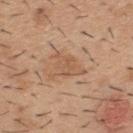| field | value |
|---|---|
| biopsy status | catalogued during a skin exam; not biopsied |
| size | about 4.5 mm |
| automated lesion analysis | an average lesion color of about L≈56 a*≈20 b*≈33 (CIELAB), a lesion–skin lightness drop of about 7, and a normalized border contrast of about 5.5; border irregularity of about 6.5 on a 0–10 scale, a color-variation rating of about 2/10, and peripheral color asymmetry of about 0.5; a lesion-detection confidence of about 100/100 |
| image source | 15 mm crop, total-body photography |
| subject | male, aged approximately 40 |
| location | the upper back |